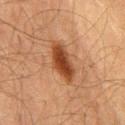Findings:
– follow-up — no biopsy performed (imaged during a skin exam)
– size — about 5 mm
– automated metrics — an average lesion color of about L≈34 a*≈21 b*≈30 (CIELAB) and about 12 CIELAB-L* units darker than the surrounding skin; border irregularity of about 3 on a 0–10 scale, internal color variation of about 3 on a 0–10 scale, and radial color variation of about 1; a nevus-likeness score of about 95/100
– location — the chest
– acquisition — 15 mm crop, total-body photography
– tile lighting — cross-polarized illumination
– patient — male, aged 58 to 62